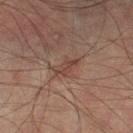{"biopsy_status": "not biopsied; imaged during a skin examination", "lighting": "cross-polarized", "image": {"source": "total-body photography crop", "field_of_view_mm": 15}, "site": "left thigh", "lesion_size": {"long_diameter_mm_approx": 3.0}, "patient": {"sex": "male", "age_approx": 75}}